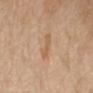Assessment:
The lesion was photographed on a routine skin check and not biopsied; there is no pathology result.
Acquisition and patient details:
Automated tile analysis of the lesion measured a shape eccentricity near 0.9. And it measured roughly 7 lightness units darker than nearby skin and a normalized lesion–skin contrast near 5.5. The software also gave border irregularity of about 4 on a 0–10 scale, a color-variation rating of about 0/10, and a peripheral color-asymmetry measure near 0. The tile uses cross-polarized illumination. Measured at roughly 3.5 mm in maximum diameter. This image is a 15 mm lesion crop taken from a total-body photograph. On the left forearm. A female patient, roughly 70 years of age.